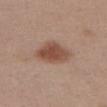workup: total-body-photography surveillance lesion; no biopsy | TBP lesion metrics: a footprint of about 12 mm² and two-axis asymmetry of about 0.25; a border-irregularity rating of about 2.5/10 and internal color variation of about 3.5 on a 0–10 scale; a nevus-likeness score of about 95/100 | tile lighting: white-light | image: 15 mm crop, total-body photography | subject: female, in their mid-50s | location: the abdomen.